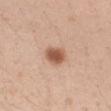notes: imaged on a skin check; not biopsied | illumination: white-light illumination | lesion diameter: ≈3 mm | patient: female, roughly 20 years of age | imaging modality: ~15 mm tile from a whole-body skin photo | automated metrics: two-axis asymmetry of about 0.15; a mean CIELAB color near L≈56 a*≈22 b*≈31, about 14 CIELAB-L* units darker than the surrounding skin, and a lesion-to-skin contrast of about 9.5 (normalized; higher = more distinct); border irregularity of about 1 on a 0–10 scale, a within-lesion color-variation index near 3/10, and a peripheral color-asymmetry measure near 0.5; an automated nevus-likeness rating near 100 out of 100 and a detector confidence of about 100 out of 100 that the crop contains a lesion | site: the right upper arm.